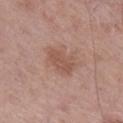notes: imaged on a skin check; not biopsied | tile lighting: white-light | subject: male, aged 78 to 82 | automated metrics: a footprint of about 7.5 mm², an outline eccentricity of about 0.75 (0 = round, 1 = elongated), and two-axis asymmetry of about 0.25 | imaging modality: 15 mm crop, total-body photography | site: the right lower leg.